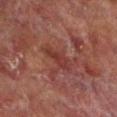follow-up: no biopsy performed (imaged during a skin exam)
illumination: cross-polarized illumination
diameter: about 4 mm
subject: male, roughly 70 years of age
site: the leg
image: 15 mm crop, total-body photography